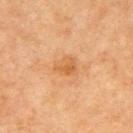The lesion was tiled from a total-body skin photograph and was not biopsied. A female patient, about 55 years old. An algorithmic analysis of the crop reported a lesion area of about 4.5 mm², a shape eccentricity near 0.55, and a symmetry-axis asymmetry near 0.4. It also reported border irregularity of about 3 on a 0–10 scale, a within-lesion color-variation index near 3/10, and radial color variation of about 1. The analysis additionally found a classifier nevus-likeness of about 0/100. The lesion is located on the left upper arm. A region of skin cropped from a whole-body photographic capture, roughly 15 mm wide. The lesion's longest dimension is about 2.5 mm.